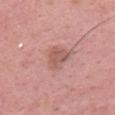Clinical impression:
No biopsy was performed on this lesion — it was imaged during a full skin examination and was not determined to be concerning.
Context:
Cropped from a whole-body photographic skin survey; the tile spans about 15 mm. The lesion is on the head or neck. A female patient, aged 48–52. Imaged with white-light lighting.